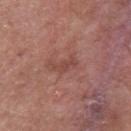Imaged during a routine full-body skin examination; the lesion was not biopsied and no histopathology is available.
A male patient aged 48–52.
An algorithmic analysis of the crop reported a shape eccentricity near 0.9 and a shape-asymmetry score of about 0.45 (0 = symmetric). And it measured a color-variation rating of about 0/10 and radial color variation of about 0.
A close-up tile cropped from a whole-body skin photograph, about 15 mm across.
Imaged with white-light lighting.
About 3 mm across.
From the mid back.